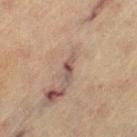Q: Was a biopsy performed?
A: no biopsy performed (imaged during a skin exam)
Q: How was the tile lit?
A: cross-polarized illumination
Q: How was this image acquired?
A: total-body-photography crop, ~15 mm field of view
Q: Patient demographics?
A: female, aged 63 to 67
Q: Where on the body is the lesion?
A: the left thigh